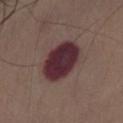{
  "biopsy_status": "not biopsied; imaged during a skin examination",
  "image": {
    "source": "total-body photography crop",
    "field_of_view_mm": 15
  },
  "lighting": "white-light",
  "site": "front of the torso",
  "patient": {
    "sex": "male",
    "age_approx": 75
  }
}